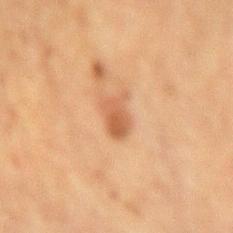Assessment:
The lesion was tiled from a total-body skin photograph and was not biopsied.
Image and clinical context:
Approximately 2.5 mm at its widest. The lesion is located on the right upper arm. A lesion tile, about 15 mm wide, cut from a 3D total-body photograph. Captured under cross-polarized illumination. A female patient roughly 50 years of age.